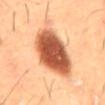biopsy status — no biopsy performed (imaged during a skin exam) | patient — male, roughly 60 years of age | size — about 8.5 mm | imaging modality — ~15 mm tile from a whole-body skin photo | body site — the abdomen | lighting — cross-polarized illumination.